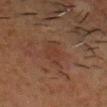Clinical impression: No biopsy was performed on this lesion — it was imaged during a full skin examination and was not determined to be concerning. Image and clinical context: Cropped from a total-body skin-imaging series; the visible field is about 15 mm. On the head or neck. The patient is a male aged approximately 60. Automated tile analysis of the lesion measured a color-variation rating of about 2/10 and peripheral color asymmetry of about 0.5. This is a cross-polarized tile. The lesion's longest dimension is about 4 mm.